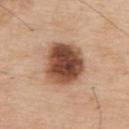biopsy status = catalogued during a skin exam; not biopsied
imaging modality = 15 mm crop, total-body photography
location = the back
subject = male, approximately 75 years of age